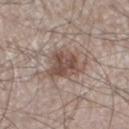Assessment:
Captured during whole-body skin photography for melanoma surveillance; the lesion was not biopsied.
Background:
Cropped from a total-body skin-imaging series; the visible field is about 15 mm. Located on the left lower leg. A male subject, roughly 60 years of age.